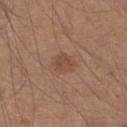Recorded during total-body skin imaging; not selected for excision or biopsy.